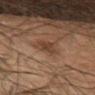Q: Is there a histopathology result?
A: total-body-photography surveillance lesion; no biopsy
Q: Lesion location?
A: the arm
Q: What did automated image analysis measure?
A: an area of roughly 4.5 mm², an eccentricity of roughly 0.75, and a symmetry-axis asymmetry near 0.3; a lesion color around L≈35 a*≈17 b*≈26 in CIELAB, a lesion–skin lightness drop of about 7, and a lesion-to-skin contrast of about 7 (normalized; higher = more distinct)
Q: Who is the patient?
A: male, approximately 60 years of age
Q: What kind of image is this?
A: ~15 mm crop, total-body skin-cancer survey
Q: What is the lesion's diameter?
A: ≈3 mm
Q: Illumination type?
A: cross-polarized